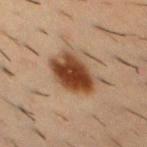Findings:
– follow-up · catalogued during a skin exam; not biopsied
– patient · male, aged around 55
– automated metrics · a mean CIELAB color near L≈37 a*≈19 b*≈30, a lesion–skin lightness drop of about 15, and a normalized lesion–skin contrast near 13; an automated nevus-likeness rating near 100 out of 100 and lesion-presence confidence of about 100/100
– site · the mid back
– lesion diameter · ≈5.5 mm
– acquisition · ~15 mm crop, total-body skin-cancer survey